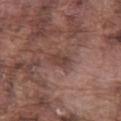Image and clinical context:
The subject is a male aged approximately 75. On the left thigh. Imaged with white-light lighting. A lesion tile, about 15 mm wide, cut from a 3D total-body photograph.